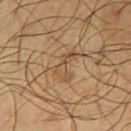The lesion was tiled from a total-body skin photograph and was not biopsied. A 15 mm close-up extracted from a 3D total-body photography capture. A male subject, approximately 65 years of age. Approximately 4 mm at its widest. The lesion is located on the left thigh. The tile uses cross-polarized illumination.